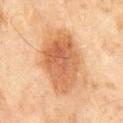biopsy_status: not biopsied; imaged during a skin examination
patient:
  sex: male
  age_approx: 45
lesion_size:
  long_diameter_mm_approx: 7.5
lighting: cross-polarized
site: mid back
image:
  source: total-body photography crop
  field_of_view_mm: 15